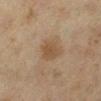workup: catalogued during a skin exam; not biopsied
subject: female, about 40 years old
location: the left lower leg
lesion size: ≈3.5 mm
imaging modality: ~15 mm tile from a whole-body skin photo
illumination: cross-polarized illumination